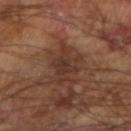Clinical impression: Recorded during total-body skin imaging; not selected for excision or biopsy. Acquisition and patient details: From the left arm. This image is a 15 mm lesion crop taken from a total-body photograph. The total-body-photography lesion software estimated an average lesion color of about L≈32 a*≈18 b*≈25 (CIELAB), about 8 CIELAB-L* units darker than the surrounding skin, and a lesion-to-skin contrast of about 7.5 (normalized; higher = more distinct). The analysis additionally found a nevus-likeness score of about 0/100 and a lesion-detection confidence of about 95/100. The subject is a male aged approximately 65. Longest diameter approximately 5 mm.